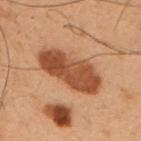workup: no biopsy performed (imaged during a skin exam) | image source: ~15 mm crop, total-body skin-cancer survey | diameter: ≈7 mm | patient: male, aged around 55 | automated metrics: a footprint of about 20 mm², an eccentricity of roughly 0.85, and a shape-asymmetry score of about 0.15 (0 = symmetric); a lesion color around L≈39 a*≈21 b*≈30 in CIELAB, roughly 12 lightness units darker than nearby skin, and a lesion-to-skin contrast of about 10 (normalized; higher = more distinct); a border-irregularity index near 2/10 and a peripheral color-asymmetry measure near 1; an automated nevus-likeness rating near 20 out of 100 and a lesion-detection confidence of about 100/100 | lighting: cross-polarized illumination | body site: the left upper arm.